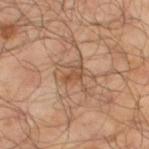workup — total-body-photography surveillance lesion; no biopsy
site — the left lower leg
acquisition — ~15 mm tile from a whole-body skin photo
patient — male, roughly 65 years of age